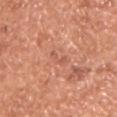Case summary:
- biopsy status · no biopsy performed (imaged during a skin exam)
- patient · male, aged approximately 65
- automated metrics · a lesion-to-skin contrast of about 5 (normalized; higher = more distinct); a nevus-likeness score of about 0/100 and a detector confidence of about 85 out of 100 that the crop contains a lesion
- lesion size · ≈3 mm
- acquisition · 15 mm crop, total-body photography
- illumination · white-light illumination
- anatomic site · the arm